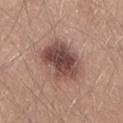Clinical impression:
The lesion was tiled from a total-body skin photograph and was not biopsied.
Background:
On the back. Automated image analysis of the tile measured a border-irregularity index near 2.5/10 and radial color variation of about 1.5. A 15 mm crop from a total-body photograph taken for skin-cancer surveillance. The patient is a male aged around 25. Longest diameter approximately 5.5 mm. Captured under white-light illumination.